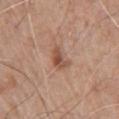Impression: No biopsy was performed on this lesion — it was imaged during a full skin examination and was not determined to be concerning. Context: The lesion is located on the chest. A lesion tile, about 15 mm wide, cut from a 3D total-body photograph. About 3 mm across. A male patient about 60 years old.